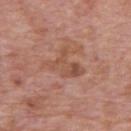This lesion was catalogued during total-body skin photography and was not selected for biopsy. Measured at roughly 5.5 mm in maximum diameter. A male subject aged approximately 70. From the upper back. A roughly 15 mm field-of-view crop from a total-body skin photograph.